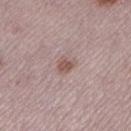<lesion>
<biopsy_status>not biopsied; imaged during a skin examination</biopsy_status>
<site>left lower leg</site>
<patient>
  <sex>female</sex>
  <age_approx>50</age_approx>
</patient>
<image>
  <source>total-body photography crop</source>
  <field_of_view_mm>15</field_of_view_mm>
</image>
</lesion>